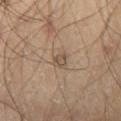biopsy_status: not biopsied; imaged during a skin examination
site: right thigh
patient:
  sex: male
  age_approx: 60
image:
  source: total-body photography crop
  field_of_view_mm: 15